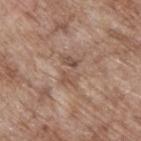Imaged during a routine full-body skin examination; the lesion was not biopsied and no histopathology is available.
The lesion-visualizer software estimated a shape eccentricity near 0.8 and a shape-asymmetry score of about 0.4 (0 = symmetric). It also reported a classifier nevus-likeness of about 0/100 and lesion-presence confidence of about 75/100.
A close-up tile cropped from a whole-body skin photograph, about 15 mm across.
The subject is a male in their 70s.
The lesion is located on the back.